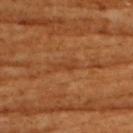Impression: No biopsy was performed on this lesion — it was imaged during a full skin examination and was not determined to be concerning. Image and clinical context: The lesion's longest dimension is about 3 mm. A female subject, in their mid-50s. A roughly 15 mm field-of-view crop from a total-body skin photograph. The lesion is on the back.